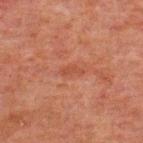A close-up tile cropped from a whole-body skin photograph, about 15 mm across. On the back. The patient is a male in their 60s. Approximately 2.5 mm at its widest. An algorithmic analysis of the crop reported an area of roughly 2 mm² and an outline eccentricity of about 0.9 (0 = round, 1 = elongated). It also reported a lesion color around L≈37 a*≈23 b*≈27 in CIELAB. The analysis additionally found a within-lesion color-variation index near 0/10 and a peripheral color-asymmetry measure near 0. This is a cross-polarized tile.